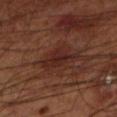biopsy status: catalogued during a skin exam; not biopsied | image-analysis metrics: a lesion area of about 8 mm² and an eccentricity of roughly 0.85; a mean CIELAB color near L≈24 a*≈21 b*≈22 and a lesion–skin lightness drop of about 6; a nevus-likeness score of about 20/100 | subject: male, aged approximately 70 | lighting: cross-polarized illumination | imaging modality: 15 mm crop, total-body photography | site: the left lower leg.